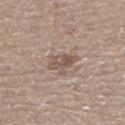{"biopsy_status": "not biopsied; imaged during a skin examination", "lighting": "white-light", "patient": {"sex": "female", "age_approx": 55}, "image": {"source": "total-body photography crop", "field_of_view_mm": 15}, "lesion_size": {"long_diameter_mm_approx": 3.5}, "site": "left lower leg"}